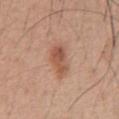{
  "biopsy_status": "not biopsied; imaged during a skin examination",
  "site": "abdomen",
  "lesion_size": {
    "long_diameter_mm_approx": 4.0
  },
  "patient": {
    "sex": "male",
    "age_approx": 55
  },
  "automated_metrics": {
    "area_mm2_approx": 7.0,
    "border_irregularity_0_10": 2.0,
    "color_variation_0_10": 4.5,
    "peripheral_color_asymmetry": 1.5
  },
  "image": {
    "source": "total-body photography crop",
    "field_of_view_mm": 15
  },
  "lighting": "white-light"
}